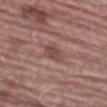Impression: This lesion was catalogued during total-body skin photography and was not selected for biopsy. Acquisition and patient details: A 15 mm crop from a total-body photograph taken for skin-cancer surveillance. Approximately 3.5 mm at its widest. Captured under white-light illumination. A male subject, aged 68 to 72. The total-body-photography lesion software estimated a lesion area of about 5 mm² and an eccentricity of roughly 0.8. It also reported about 9 CIELAB-L* units darker than the surrounding skin and a normalized lesion–skin contrast near 7. On the leg.